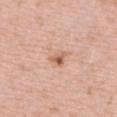| feature | finding |
|---|---|
| follow-up | no biopsy performed (imaged during a skin exam) |
| subject | female, roughly 40 years of age |
| imaging modality | ~15 mm tile from a whole-body skin photo |
| body site | the chest |